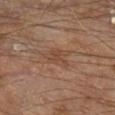follow-up: imaged on a skin check; not biopsied
lesion diameter: ≈3.5 mm
patient: male, approximately 70 years of age
image-analysis metrics: an area of roughly 5.5 mm² and an outline eccentricity of about 0.7 (0 = round, 1 = elongated); a lesion color around L≈44 a*≈18 b*≈29 in CIELAB and a normalized lesion–skin contrast near 5.5; border irregularity of about 4.5 on a 0–10 scale, a within-lesion color-variation index near 1.5/10, and radial color variation of about 0.5; an automated nevus-likeness rating near 0 out of 100 and a lesion-detection confidence of about 100/100
site: the right lower leg
image source: total-body-photography crop, ~15 mm field of view